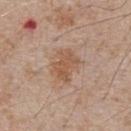Clinical impression:
Imaged during a routine full-body skin examination; the lesion was not biopsied and no histopathology is available.
Image and clinical context:
Located on the chest. This is a white-light tile. Automated tile analysis of the lesion measured lesion-presence confidence of about 100/100. This image is a 15 mm lesion crop taken from a total-body photograph. A male subject in their mid-60s.